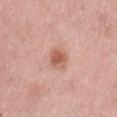The lesion was photographed on a routine skin check and not biopsied; there is no pathology result.
The subject is a female aged 38 to 42.
Captured under white-light illumination.
This image is a 15 mm lesion crop taken from a total-body photograph.
Approximately 3 mm at its widest.
The lesion is on the left thigh.